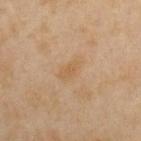Q: Lesion size?
A: ~3.5 mm (longest diameter)
Q: Automated lesion metrics?
A: a footprint of about 6 mm², an eccentricity of roughly 0.8, and two-axis asymmetry of about 0.35; an average lesion color of about L≈57 a*≈15 b*≈35 (CIELAB), about 5 CIELAB-L* units darker than the surrounding skin, and a normalized lesion–skin contrast near 4.5; a border-irregularity rating of about 3/10 and internal color variation of about 2.5 on a 0–10 scale
Q: Patient demographics?
A: female, about 35 years old
Q: How was this image acquired?
A: ~15 mm tile from a whole-body skin photo
Q: Lesion location?
A: the upper back